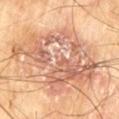• follow-up · catalogued during a skin exam; not biopsied
• body site · the left thigh
• size · ~12 mm (longest diameter)
• automated lesion analysis · an area of roughly 60 mm², an eccentricity of roughly 0.7, and a symmetry-axis asymmetry near 0.3; roughly 11 lightness units darker than nearby skin and a normalized border contrast of about 7; a border-irregularity rating of about 6/10, a color-variation rating of about 8.5/10, and a peripheral color-asymmetry measure near 2.5
• image · ~15 mm crop, total-body skin-cancer survey
• subject · male, aged around 70